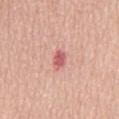workup: no biopsy performed (imaged during a skin exam) | size: ≈2.5 mm | illumination: white-light | anatomic site: the mid back | automated metrics: an eccentricity of roughly 0.75 and a shape-asymmetry score of about 0.15 (0 = symmetric) | image: 15 mm crop, total-body photography | subject: female, aged 63–67.